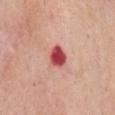Context:
A 15 mm close-up extracted from a 3D total-body photography capture. A male subject, roughly 65 years of age. An algorithmic analysis of the crop reported a lesion area of about 6 mm² and a symmetry-axis asymmetry near 0.15. And it measured an average lesion color of about L≈52 a*≈38 b*≈26 (CIELAB) and roughly 18 lightness units darker than nearby skin. It also reported border irregularity of about 1.5 on a 0–10 scale and internal color variation of about 3.5 on a 0–10 scale. And it measured a classifier nevus-likeness of about 0/100. The lesion is on the chest. Approximately 3 mm at its widest. This is a white-light tile.